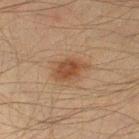Notes:
- image — 15 mm crop, total-body photography
- subject — male, aged 48 to 52
- location — the left thigh
- diameter — ≈4 mm
- illumination — cross-polarized